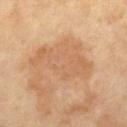Notes:
– workup: imaged on a skin check; not biopsied
– diameter: ~7.5 mm (longest diameter)
– image: 15 mm crop, total-body photography
– automated lesion analysis: a shape eccentricity near 0.9 and a symmetry-axis asymmetry near 0.35; a mean CIELAB color near L≈60 a*≈20 b*≈35 and about 7 CIELAB-L* units darker than the surrounding skin; a border-irregularity index near 6/10, internal color variation of about 3 on a 0–10 scale, and peripheral color asymmetry of about 1; a classifier nevus-likeness of about 5/100
– illumination: cross-polarized illumination
– patient: male, aged around 65